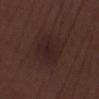notes = no biopsy performed (imaged during a skin exam)
lesion size = ~5 mm (longest diameter)
image = total-body-photography crop, ~15 mm field of view
lighting = white-light
anatomic site = the right lower leg
patient = male, aged around 70